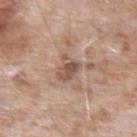No biopsy was performed on this lesion — it was imaged during a full skin examination and was not determined to be concerning.
Imaged with white-light lighting.
The patient is a male in their mid-70s.
Located on the left upper arm.
Automated image analysis of the tile measured a footprint of about 6 mm² and a symmetry-axis asymmetry near 0.35. The software also gave border irregularity of about 3.5 on a 0–10 scale, a within-lesion color-variation index near 3.5/10, and a peripheral color-asymmetry measure near 1. The software also gave a classifier nevus-likeness of about 0/100.
A roughly 15 mm field-of-view crop from a total-body skin photograph.
Measured at roughly 3 mm in maximum diameter.